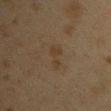Q: Is there a histopathology result?
A: catalogued during a skin exam; not biopsied
Q: What is the anatomic site?
A: the arm
Q: Who is the patient?
A: female, about 40 years old
Q: Automated lesion metrics?
A: an area of roughly 3.5 mm², an eccentricity of roughly 0.9, and a shape-asymmetry score of about 0.5 (0 = symmetric); border irregularity of about 6.5 on a 0–10 scale, internal color variation of about 0.5 on a 0–10 scale, and peripheral color asymmetry of about 0; a classifier nevus-likeness of about 0/100 and a lesion-detection confidence of about 100/100
Q: What kind of image is this?
A: 15 mm crop, total-body photography
Q: Illumination type?
A: cross-polarized illumination